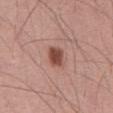This lesion was catalogued during total-body skin photography and was not selected for biopsy. The lesion is on the front of the torso. The subject is a male in their mid-50s. Measured at roughly 3 mm in maximum diameter. This image is a 15 mm lesion crop taken from a total-body photograph. This is a white-light tile.